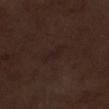Part of a total-body skin-imaging series; this lesion was reviewed on a skin check and was not flagged for biopsy.
The patient is a male in their 70s.
The lesion is on the left lower leg.
This image is a 15 mm lesion crop taken from a total-body photograph.